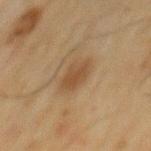- workup: imaged on a skin check; not biopsied
- imaging modality: total-body-photography crop, ~15 mm field of view
- patient: male, aged 68–72
- anatomic site: the mid back
- automated metrics: a lesion color around L≈40 a*≈15 b*≈29 in CIELAB and about 7 CIELAB-L* units darker than the surrounding skin